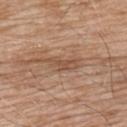Part of a total-body skin-imaging series; this lesion was reviewed on a skin check and was not flagged for biopsy. This image is a 15 mm lesion crop taken from a total-body photograph. From the upper back. The patient is a male aged around 60.